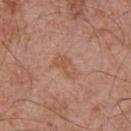Findings:
- workup · total-body-photography surveillance lesion; no biopsy
- illumination · white-light illumination
- subject · male, about 70 years old
- imaging modality · total-body-photography crop, ~15 mm field of view
- size · about 3.5 mm
- site · the chest